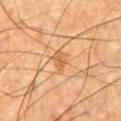workup — total-body-photography surveillance lesion; no biopsy | body site — the chest | automated lesion analysis — an eccentricity of roughly 0.8 and two-axis asymmetry of about 0.3; a mean CIELAB color near L≈59 a*≈22 b*≈42, roughly 8 lightness units darker than nearby skin, and a lesion-to-skin contrast of about 6 (normalized; higher = more distinct); border irregularity of about 3 on a 0–10 scale; a classifier nevus-likeness of about 0/100 | patient — male, aged around 65 | image — ~15 mm crop, total-body skin-cancer survey | diameter — ~3 mm (longest diameter).